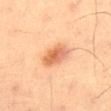Q: Is there a histopathology result?
A: catalogued during a skin exam; not biopsied
Q: What lighting was used for the tile?
A: cross-polarized illumination
Q: What is the anatomic site?
A: the leg
Q: Lesion size?
A: ≈3.5 mm
Q: What kind of image is this?
A: 15 mm crop, total-body photography
Q: Automated lesion metrics?
A: a lesion–skin lightness drop of about 11 and a normalized border contrast of about 7.5; a border-irregularity rating of about 2/10 and a peripheral color-asymmetry measure near 2; a detector confidence of about 100 out of 100 that the crop contains a lesion
Q: Patient demographics?
A: male, about 55 years old